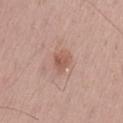workup: catalogued during a skin exam; not biopsied | patient: male, aged approximately 55 | anatomic site: the lower back | tile lighting: white-light illumination | acquisition: 15 mm crop, total-body photography.